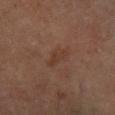This lesion was catalogued during total-body skin photography and was not selected for biopsy. Located on the left lower leg. A male patient aged 58 to 62. A 15 mm crop from a total-body photograph taken for skin-cancer surveillance. The lesion's longest dimension is about 4 mm. Imaged with cross-polarized lighting.